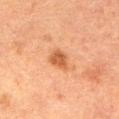Impression: This lesion was catalogued during total-body skin photography and was not selected for biopsy. Background: Measured at roughly 2.5 mm in maximum diameter. Imaged with cross-polarized lighting. The subject is a female about 50 years old. On the front of the torso. A 15 mm close-up tile from a total-body photography series done for melanoma screening.